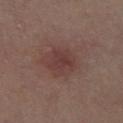Acquisition and patient details: Cropped from a total-body skin-imaging series; the visible field is about 15 mm. About 3.5 mm across. On the leg. A female subject in their 50s.